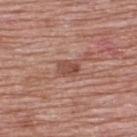– notes · catalogued during a skin exam; not biopsied